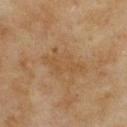Part of a total-body skin-imaging series; this lesion was reviewed on a skin check and was not flagged for biopsy.
This is a cross-polarized tile.
A 15 mm close-up extracted from a 3D total-body photography capture.
The total-body-photography lesion software estimated a lesion area of about 10 mm² and an outline eccentricity of about 0.9 (0 = round, 1 = elongated). The analysis additionally found an average lesion color of about L≈47 a*≈16 b*≈34 (CIELAB), about 6 CIELAB-L* units darker than the surrounding skin, and a lesion-to-skin contrast of about 5 (normalized; higher = more distinct). The analysis additionally found a border-irregularity index near 5/10, a color-variation rating of about 2/10, and a peripheral color-asymmetry measure near 0.5. The software also gave a nevus-likeness score of about 0/100 and lesion-presence confidence of about 100/100.
The lesion is on the upper back.
A female patient aged around 60.
Measured at roughly 5.5 mm in maximum diameter.